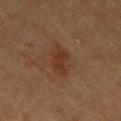Assessment:
Captured during whole-body skin photography for melanoma surveillance; the lesion was not biopsied.
Clinical summary:
The lesion is on the chest. This is a cross-polarized tile. A female patient roughly 65 years of age. A roughly 15 mm field-of-view crop from a total-body skin photograph.